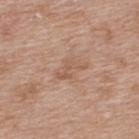Assessment:
Recorded during total-body skin imaging; not selected for excision or biopsy.
Background:
The subject is a female aged around 40. About 4 mm across. A region of skin cropped from a whole-body photographic capture, roughly 15 mm wide. On the upper back. Captured under white-light illumination.